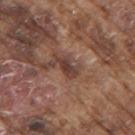follow-up=imaged on a skin check; not biopsied | patient=male, aged 73 to 77 | image source=total-body-photography crop, ~15 mm field of view | site=the arm.